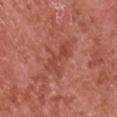Clinical impression:
Imaged during a routine full-body skin examination; the lesion was not biopsied and no histopathology is available.
Background:
This is a white-light tile. The subject is a male aged 63–67. Approximately 4.5 mm at its widest. Located on the left upper arm. This image is a 15 mm lesion crop taken from a total-body photograph.